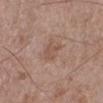No biopsy was performed on this lesion — it was imaged during a full skin examination and was not determined to be concerning. On the left lower leg. A close-up tile cropped from a whole-body skin photograph, about 15 mm across. A male subject, aged around 50.